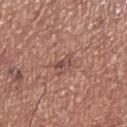Impression: The lesion was photographed on a routine skin check and not biopsied; there is no pathology result. Image and clinical context: From the right lower leg. Automated image analysis of the tile measured an area of roughly 2.5 mm², an eccentricity of roughly 0.85, and two-axis asymmetry of about 0.55. And it measured a within-lesion color-variation index near 0/10 and a peripheral color-asymmetry measure near 0. Measured at roughly 2.5 mm in maximum diameter. This image is a 15 mm lesion crop taken from a total-body photograph. A male subject aged around 70. Imaged with white-light lighting.